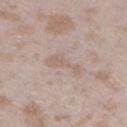biopsy status: catalogued during a skin exam; not biopsied
image-analysis metrics: an eccentricity of roughly 0.95 and a shape-asymmetry score of about 0.55 (0 = symmetric); a mean CIELAB color near L≈61 a*≈14 b*≈24, a lesion–skin lightness drop of about 6, and a normalized lesion–skin contrast near 4.5
illumination: white-light illumination
body site: the leg
image: 15 mm crop, total-body photography
subject: female, aged approximately 25